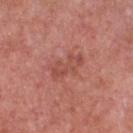Recorded during total-body skin imaging; not selected for excision or biopsy. Captured under white-light illumination. The lesion's longest dimension is about 4 mm. Cropped from a whole-body photographic skin survey; the tile spans about 15 mm. A male patient aged 68–72. On the chest.